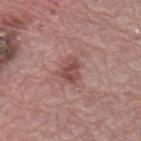follow-up — total-body-photography surveillance lesion; no biopsy | anatomic site — the mid back | subject — male, approximately 70 years of age | diameter — about 3 mm | imaging modality — ~15 mm tile from a whole-body skin photo | tile lighting — white-light | TBP lesion metrics — an outline eccentricity of about 0.65 (0 = round, 1 = elongated) and a symmetry-axis asymmetry near 0.3; an average lesion color of about L≈49 a*≈24 b*≈23 (CIELAB), about 10 CIELAB-L* units darker than the surrounding skin, and a lesion-to-skin contrast of about 7 (normalized; higher = more distinct); border irregularity of about 3 on a 0–10 scale, a within-lesion color-variation index near 4.5/10, and peripheral color asymmetry of about 2.